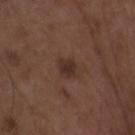The subject is a female aged around 35. The lesion's longest dimension is about 3 mm. An algorithmic analysis of the crop reported a lesion area of about 4 mm², an outline eccentricity of about 0.75 (0 = round, 1 = elongated), and a shape-asymmetry score of about 0.2 (0 = symmetric). The software also gave a lesion color around L≈30 a*≈17 b*≈21 in CIELAB, a lesion–skin lightness drop of about 8, and a normalized border contrast of about 8. It also reported border irregularity of about 2 on a 0–10 scale, a color-variation rating of about 1.5/10, and peripheral color asymmetry of about 0.5. The lesion is located on the upper back. A 15 mm close-up tile from a total-body photography series done for melanoma screening.